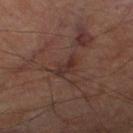  biopsy_status: not biopsied; imaged during a skin examination
  image:
    source: total-body photography crop
    field_of_view_mm: 15
  lighting: cross-polarized
  patient:
    sex: male
    age_approx: 70
  lesion_size:
    long_diameter_mm_approx: 3.0
  site: leg